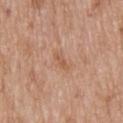Q: Is there a histopathology result?
A: no biopsy performed (imaged during a skin exam)
Q: How was this image acquired?
A: ~15 mm tile from a whole-body skin photo
Q: What are the patient's age and sex?
A: male, aged 78 to 82
Q: Where on the body is the lesion?
A: the upper back